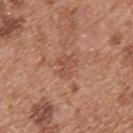biopsy status: total-body-photography surveillance lesion; no biopsy.